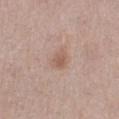Notes:
- notes: no biopsy performed (imaged during a skin exam)